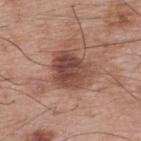Notes:
– biopsy status: no biopsy performed (imaged during a skin exam)
– diameter: ≈4.5 mm
– patient: male, aged 68 to 72
– location: the upper back
– tile lighting: white-light
– imaging modality: total-body-photography crop, ~15 mm field of view